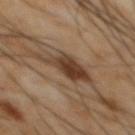- biopsy status · imaged on a skin check; not biopsied
- tile lighting · cross-polarized
- image source · 15 mm crop, total-body photography
- image-analysis metrics · a lesion color around L≈37 a*≈16 b*≈26 in CIELAB and a normalized lesion–skin contrast near 9.5; a nevus-likeness score of about 75/100
- site · the abdomen
- subject · male, aged 63 to 67